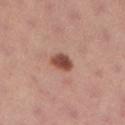Clinical impression:
Part of a total-body skin-imaging series; this lesion was reviewed on a skin check and was not flagged for biopsy.
Acquisition and patient details:
The lesion is located on the right lower leg. The lesion-visualizer software estimated a footprint of about 5 mm². The analysis additionally found a border-irregularity index near 1.5/10, a color-variation rating of about 4/10, and peripheral color asymmetry of about 1.5. It also reported a lesion-detection confidence of about 100/100. The recorded lesion diameter is about 3 mm. The patient is a female aged 23–27. A region of skin cropped from a whole-body photographic capture, roughly 15 mm wide.